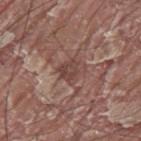biopsy status — total-body-photography surveillance lesion; no biopsy | image source — total-body-photography crop, ~15 mm field of view | lesion size — ~3 mm (longest diameter) | automated lesion analysis — a shape eccentricity near 0.45 and two-axis asymmetry of about 0.4; a lesion color around L≈44 a*≈19 b*≈23 in CIELAB and a lesion-to-skin contrast of about 7 (normalized; higher = more distinct); an automated nevus-likeness rating near 0 out of 100 and lesion-presence confidence of about 80/100 | subject — male, aged 38 to 42 | illumination — white-light | location — the mid back.